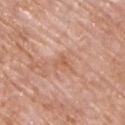size — ≈2.5 mm | image source — 15 mm crop, total-body photography | tile lighting — white-light illumination | anatomic site — the chest | subject — male, aged 58 to 62 | automated lesion analysis — an area of roughly 3 mm² and a shape-asymmetry score of about 0.6 (0 = symmetric); lesion-presence confidence of about 100/100.